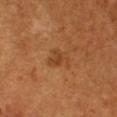Notes:
– workup · no biopsy performed (imaged during a skin exam)
– image source · 15 mm crop, total-body photography
– tile lighting · cross-polarized
– size · about 3 mm
– subject · female, roughly 55 years of age
– TBP lesion metrics · an area of roughly 5.5 mm² and a symmetry-axis asymmetry near 0.35; a lesion color around L≈45 a*≈24 b*≈39 in CIELAB, about 7 CIELAB-L* units darker than the surrounding skin, and a lesion-to-skin contrast of about 5.5 (normalized; higher = more distinct)
– anatomic site · the chest